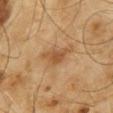Assessment: Captured during whole-body skin photography for melanoma surveillance; the lesion was not biopsied. Image and clinical context: Located on the mid back. This image is a 15 mm lesion crop taken from a total-body photograph. A male subject, in their mid-60s.